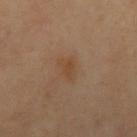biopsy status = imaged on a skin check; not biopsied.